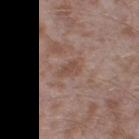Q: Illumination type?
A: white-light illumination
Q: Lesion size?
A: ≈3 mm
Q: What are the patient's age and sex?
A: male, aged approximately 45
Q: Where on the body is the lesion?
A: the right thigh
Q: What did automated image analysis measure?
A: a border-irregularity index near 3/10 and a color-variation rating of about 1.5/10; an automated nevus-likeness rating near 0 out of 100 and lesion-presence confidence of about 95/100
Q: What is the imaging modality?
A: 15 mm crop, total-body photography